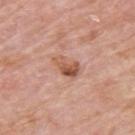follow-up = no biopsy performed (imaged during a skin exam); size = ≈3 mm; imaging modality = 15 mm crop, total-body photography; site = the upper back; patient = male, approximately 75 years of age.